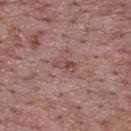Clinical impression:
Recorded during total-body skin imaging; not selected for excision or biopsy.